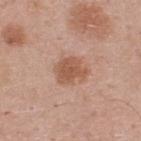The lesion was photographed on a routine skin check and not biopsied; there is no pathology result. Automated tile analysis of the lesion measured radial color variation of about 0.5. It also reported a classifier nevus-likeness of about 30/100 and a detector confidence of about 100 out of 100 that the crop contains a lesion. From the upper back. The subject is a male about 45 years old. Approximately 3.5 mm at its widest. Cropped from a whole-body photographic skin survey; the tile spans about 15 mm.